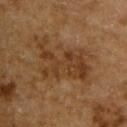Impression:
The lesion was photographed on a routine skin check and not biopsied; there is no pathology result.
Context:
The lesion is located on the back. A close-up tile cropped from a whole-body skin photograph, about 15 mm across. A male subject roughly 65 years of age.